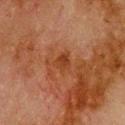Case summary:
• image: ~15 mm tile from a whole-body skin photo
• illumination: cross-polarized illumination
• lesion size: about 2.5 mm
• site: the upper back
• patient: male, roughly 80 years of age